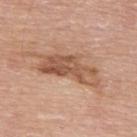* biopsy status · imaged on a skin check; not biopsied
* lesion size · ≈7 mm
* patient · male, aged approximately 80
* image · total-body-photography crop, ~15 mm field of view
* body site · the upper back
* automated lesion analysis · a border-irregularity rating of about 4.5/10, internal color variation of about 6.5 on a 0–10 scale, and peripheral color asymmetry of about 2.5; a nevus-likeness score of about 45/100 and a lesion-detection confidence of about 100/100